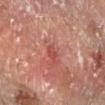follow-up: imaged on a skin check; not biopsied | patient: male, in their 60s | size: about 2 mm | automated lesion analysis: a footprint of about 2.5 mm², a shape eccentricity near 0.55, and a shape-asymmetry score of about 0.3 (0 = symmetric) | site: the right forearm | imaging modality: 15 mm crop, total-body photography.